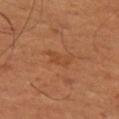<lesion>
<biopsy_status>not biopsied; imaged during a skin examination</biopsy_status>
<site>left thigh</site>
<patient>
  <sex>male</sex>
  <age_approx>50</age_approx>
</patient>
<lesion_size>
  <long_diameter_mm_approx>3.5</long_diameter_mm_approx>
</lesion_size>
<lighting>cross-polarized</lighting>
<image>
  <source>total-body photography crop</source>
  <field_of_view_mm>15</field_of_view_mm>
</image>
</lesion>